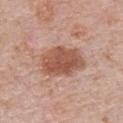Impression:
This lesion was catalogued during total-body skin photography and was not selected for biopsy.
Clinical summary:
A male patient aged 68 to 72. Located on the chest. Measured at roughly 5.5 mm in maximum diameter. A region of skin cropped from a whole-body photographic capture, roughly 15 mm wide. This is a white-light tile.